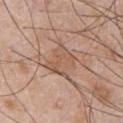No biopsy was performed on this lesion — it was imaged during a full skin examination and was not determined to be concerning. The total-body-photography lesion software estimated a border-irregularity rating of about 6.5/10, a within-lesion color-variation index near 2/10, and peripheral color asymmetry of about 0.5. Approximately 4 mm at its widest. The patient is a male in their 50s. Located on the front of the torso. This is a white-light tile. Cropped from a whole-body photographic skin survey; the tile spans about 15 mm.